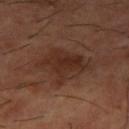Findings:
• workup · no biopsy performed (imaged during a skin exam)
• lesion diameter · about 5 mm
• site · the right thigh
• image · ~15 mm tile from a whole-body skin photo
• patient · male, in their mid-60s
• tile lighting · cross-polarized